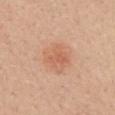Notes:
• biopsy status — no biopsy performed (imaged during a skin exam)
• lighting — white-light illumination
• lesion size — ≈4 mm
• patient — female, in their mid- to late 40s
• body site — the upper back
• TBP lesion metrics — a nevus-likeness score of about 60/100
• image source — 15 mm crop, total-body photography